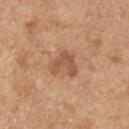Q: Was this lesion biopsied?
A: catalogued during a skin exam; not biopsied
Q: Patient demographics?
A: male, roughly 60 years of age
Q: Lesion location?
A: the head or neck
Q: How was the tile lit?
A: white-light illumination
Q: What kind of image is this?
A: total-body-photography crop, ~15 mm field of view
Q: What did automated image analysis measure?
A: border irregularity of about 6 on a 0–10 scale, a color-variation rating of about 2/10, and peripheral color asymmetry of about 0.5; an automated nevus-likeness rating near 0 out of 100